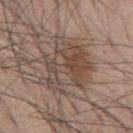Notes:
• follow-up · imaged on a skin check; not biopsied
• site · the mid back
• acquisition · 15 mm crop, total-body photography
• illumination · white-light illumination
• patient · male, aged 43–47
• lesion size · ~6.5 mm (longest diameter)
• automated lesion analysis · a peripheral color-asymmetry measure near 2.5; a classifier nevus-likeness of about 60/100